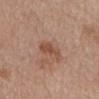follow-up: imaged on a skin check; not biopsied
lesion size: ~4 mm (longest diameter)
body site: the mid back
automated metrics: a lesion color around L≈50 a*≈21 b*≈30 in CIELAB and roughly 9 lightness units darker than nearby skin
patient: male, approximately 80 years of age
image: ~15 mm tile from a whole-body skin photo
illumination: white-light illumination